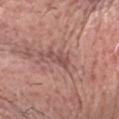Q: Was a biopsy performed?
A: total-body-photography surveillance lesion; no biopsy
Q: Lesion size?
A: ≈3.5 mm
Q: Lesion location?
A: the head or neck
Q: How was the tile lit?
A: white-light
Q: Who is the patient?
A: male, in their mid- to late 70s
Q: What is the imaging modality?
A: ~15 mm tile from a whole-body skin photo
Q: What did automated image analysis measure?
A: a border-irregularity index near 4/10, a within-lesion color-variation index near 1.5/10, and radial color variation of about 0.5; a nevus-likeness score of about 0/100 and lesion-presence confidence of about 85/100